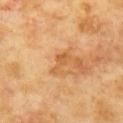The lesion was photographed on a routine skin check and not biopsied; there is no pathology result.
On the chest.
Approximately 3 mm at its widest.
Captured under cross-polarized illumination.
The total-body-photography lesion software estimated an eccentricity of roughly 0.9 and a symmetry-axis asymmetry near 0.55. The software also gave a classifier nevus-likeness of about 0/100 and a lesion-detection confidence of about 100/100.
This image is a 15 mm lesion crop taken from a total-body photograph.
A male subject aged 58 to 62.